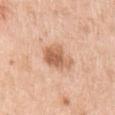Assessment: Recorded during total-body skin imaging; not selected for excision or biopsy. Clinical summary: Cropped from a whole-body photographic skin survey; the tile spans about 15 mm. The lesion is located on the arm. A male subject aged 68–72.